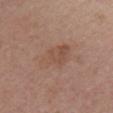workup = catalogued during a skin exam; not biopsied
subject = female, about 55 years old
site = the chest
illumination = white-light illumination
automated metrics = a border-irregularity index near 3/10, internal color variation of about 3 on a 0–10 scale, and peripheral color asymmetry of about 1; a detector confidence of about 100 out of 100 that the crop contains a lesion
acquisition = ~15 mm crop, total-body skin-cancer survey
lesion diameter = ≈4.5 mm